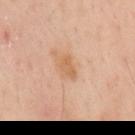Assessment: The lesion was tiled from a total-body skin photograph and was not biopsied. Context: Cropped from a total-body skin-imaging series; the visible field is about 15 mm. Automated image analysis of the tile measured a border-irregularity index near 3/10. The software also gave a classifier nevus-likeness of about 5/100 and lesion-presence confidence of about 100/100. From the mid back. Imaged with cross-polarized lighting. A male subject, aged around 55.